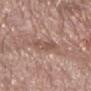follow-up: no biopsy performed (imaged during a skin exam); body site: the left forearm; image: ~15 mm tile from a whole-body skin photo; patient: male, aged 53–57; lesion size: about 3 mm; lighting: white-light illumination.